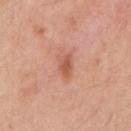Context: A female subject aged 53–57. A 15 mm crop from a total-body photograph taken for skin-cancer surveillance. From the right upper arm.